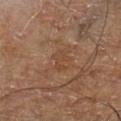Findings:
- biopsy status · imaged on a skin check; not biopsied
- tile lighting · cross-polarized illumination
- image · 15 mm crop, total-body photography
- subject · male, aged 68 to 72
- automated metrics · border irregularity of about 5.5 on a 0–10 scale, a color-variation rating of about 1/10, and radial color variation of about 0.5
- location · the left lower leg